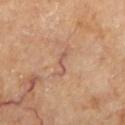Q: What is the anatomic site?
A: the left lower leg
Q: Patient demographics?
A: female, aged around 60
Q: How was the tile lit?
A: cross-polarized illumination
Q: What kind of image is this?
A: 15 mm crop, total-body photography